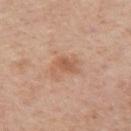{
  "lighting": "white-light",
  "site": "left upper arm",
  "lesion_size": {
    "long_diameter_mm_approx": 3.0
  },
  "image": {
    "source": "total-body photography crop",
    "field_of_view_mm": 15
  },
  "patient": {
    "sex": "male",
    "age_approx": 75
  }
}